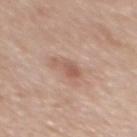Image and clinical context:
A female patient aged approximately 65. Cropped from a whole-body photographic skin survey; the tile spans about 15 mm. Captured under white-light illumination. The lesion is located on the upper back. The lesion-visualizer software estimated a lesion area of about 4.5 mm² and an outline eccentricity of about 0.8 (0 = round, 1 = elongated). It also reported a normalized border contrast of about 6. The software also gave a border-irregularity index near 5/10, a within-lesion color-variation index near 2.5/10, and peripheral color asymmetry of about 1. Measured at roughly 3 mm in maximum diameter.